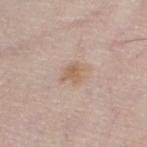lesion size: ≈3.5 mm
image-analysis metrics: a lesion–skin lightness drop of about 8 and a lesion-to-skin contrast of about 6 (normalized; higher = more distinct); border irregularity of about 3 on a 0–10 scale, a within-lesion color-variation index near 2.5/10, and a peripheral color-asymmetry measure near 0.5; an automated nevus-likeness rating near 20 out of 100 and a lesion-detection confidence of about 100/100
tile lighting: white-light illumination
patient: female, in their 60s
acquisition: 15 mm crop, total-body photography
site: the leg